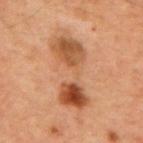Q: Was this lesion biopsied?
A: catalogued during a skin exam; not biopsied
Q: Where on the body is the lesion?
A: the chest
Q: Patient demographics?
A: male, aged approximately 60
Q: What lighting was used for the tile?
A: cross-polarized
Q: How large is the lesion?
A: ≈8.5 mm
Q: What is the imaging modality?
A: total-body-photography crop, ~15 mm field of view